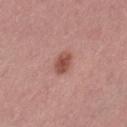Assessment:
The lesion was photographed on a routine skin check and not biopsied; there is no pathology result.
Background:
The patient is a female roughly 55 years of age. Longest diameter approximately 2.5 mm. The tile uses white-light illumination. This image is a 15 mm lesion crop taken from a total-body photograph. The lesion is on the leg.